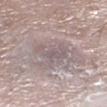Case summary:
• size: ≈4 mm
• TBP lesion metrics: an eccentricity of roughly 0.8 and two-axis asymmetry of about 0.5; an average lesion color of about L≈56 a*≈12 b*≈15 (CIELAB), roughly 7 lightness units darker than nearby skin, and a normalized border contrast of about 5; border irregularity of about 6.5 on a 0–10 scale, a within-lesion color-variation index near 1.5/10, and radial color variation of about 0.5
• patient: male, in their mid-80s or older
• lighting: white-light
• image: total-body-photography crop, ~15 mm field of view
• anatomic site: the left lower leg
• histopathology: an actinic keratosis (borderline)